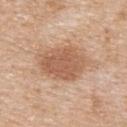  image:
    source: total-body photography crop
    field_of_view_mm: 15
  patient:
    sex: male
    age_approx: 60
  automated_metrics:
    border_irregularity_0_10: 2.0
    color_variation_0_10: 3.0
    peripheral_color_asymmetry: 1.0
  site: back
  lesion_size:
    long_diameter_mm_approx: 6.5
  lighting: white-light